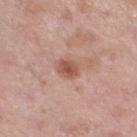Impression:
Part of a total-body skin-imaging series; this lesion was reviewed on a skin check and was not flagged for biopsy.
Background:
The total-body-photography lesion software estimated an average lesion color of about L≈54 a*≈23 b*≈28 (CIELAB) and a lesion–skin lightness drop of about 11. The software also gave a color-variation rating of about 2.5/10 and radial color variation of about 1. A female patient aged approximately 50. A lesion tile, about 15 mm wide, cut from a 3D total-body photograph. Approximately 3 mm at its widest. Captured under white-light illumination. On the left lower leg.